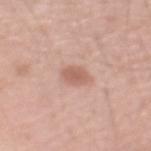Impression: Part of a total-body skin-imaging series; this lesion was reviewed on a skin check and was not flagged for biopsy. Context: Imaged with white-light lighting. From the left upper arm. A male patient, aged around 55. A close-up tile cropped from a whole-body skin photograph, about 15 mm across.